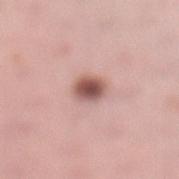Notes:
– workup: imaged on a skin check; not biopsied